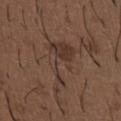* follow-up · total-body-photography surveillance lesion; no biopsy
* patient · male, aged around 50
* image source · ~15 mm tile from a whole-body skin photo
* anatomic site · the chest